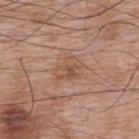Findings:
• biopsy status · total-body-photography surveillance lesion; no biopsy
• imaging modality · ~15 mm tile from a whole-body skin photo
• anatomic site · the upper back
• subject · male, approximately 70 years of age